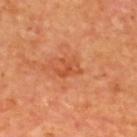Part of a total-body skin-imaging series; this lesion was reviewed on a skin check and was not flagged for biopsy. A lesion tile, about 15 mm wide, cut from a 3D total-body photograph. From the upper back. A male subject in their mid-60s. This is a cross-polarized tile. Measured at roughly 3 mm in maximum diameter.